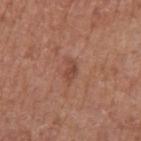The lesion was tiled from a total-body skin photograph and was not biopsied. Measured at roughly 2.5 mm in maximum diameter. Captured under white-light illumination. A male subject aged 73 to 77. A 15 mm crop from a total-body photograph taken for skin-cancer surveillance. On the arm.